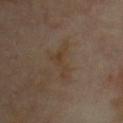lighting=cross-polarized | patient=male, aged around 65 | anatomic site=the chest | lesion diameter=≈4.5 mm | image=total-body-photography crop, ~15 mm field of view.